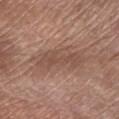<record>
<biopsy_status>not biopsied; imaged during a skin examination</biopsy_status>
<lesion_size>
  <long_diameter_mm_approx>6.0</long_diameter_mm_approx>
</lesion_size>
<lighting>white-light</lighting>
<image>
  <source>total-body photography crop</source>
  <field_of_view_mm>15</field_of_view_mm>
</image>
<automated_metrics>
  <area_mm2_approx>9.5</area_mm2_approx>
  <eccentricity>0.95</eccentricity>
  <shape_asymmetry>0.35</shape_asymmetry>
  <border_irregularity_0_10>6.5</border_irregularity_0_10>
  <color_variation_0_10>1.5</color_variation_0_10>
  <peripheral_color_asymmetry>0.5</peripheral_color_asymmetry>
  <nevus_likeness_0_100>5</nevus_likeness_0_100>
  <lesion_detection_confidence_0_100>90</lesion_detection_confidence_0_100>
</automated_metrics>
<site>left lower leg</site>
<patient>
  <sex>male</sex>
  <age_approx>70</age_approx>
</patient>
</record>